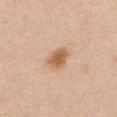biopsy status: no biopsy performed (imaged during a skin exam) | lesion diameter: about 3 mm | acquisition: ~15 mm tile from a whole-body skin photo | subject: female, aged around 55 | lighting: white-light | TBP lesion metrics: a lesion area of about 6 mm², a shape eccentricity near 0.65, and a shape-asymmetry score of about 0.2 (0 = symmetric); a mean CIELAB color near L≈61 a*≈20 b*≈35, roughly 12 lightness units darker than nearby skin, and a normalized border contrast of about 8.5; border irregularity of about 2 on a 0–10 scale and radial color variation of about 0.5 | body site: the chest.